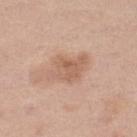Part of a total-body skin-imaging series; this lesion was reviewed on a skin check and was not flagged for biopsy. Imaged with white-light lighting. Approximately 4.5 mm at its widest. A 15 mm close-up tile from a total-body photography series done for melanoma screening. A female patient, aged approximately 55. From the left lower leg.